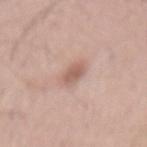Q: Was this lesion biopsied?
A: catalogued during a skin exam; not biopsied
Q: Automated lesion metrics?
A: a footprint of about 4 mm², a shape eccentricity near 0.8, and a symmetry-axis asymmetry near 0.25; a lesion color around L≈59 a*≈20 b*≈25 in CIELAB, about 11 CIELAB-L* units darker than the surrounding skin, and a normalized lesion–skin contrast near 7; a classifier nevus-likeness of about 50/100 and a detector confidence of about 100 out of 100 that the crop contains a lesion
Q: What are the patient's age and sex?
A: male, aged 53–57
Q: How was this image acquired?
A: ~15 mm tile from a whole-body skin photo
Q: What is the anatomic site?
A: the back
Q: How was the tile lit?
A: white-light illumination
Q: How large is the lesion?
A: ≈3 mm